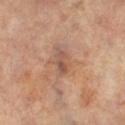Assessment:
The lesion was photographed on a routine skin check and not biopsied; there is no pathology result.
Clinical summary:
A 15 mm crop from a total-body photograph taken for skin-cancer surveillance. The lesion is on the left leg. A female patient about 65 years old.